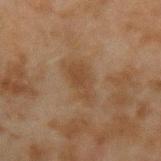The lesion was tiled from a total-body skin photograph and was not biopsied. A 15 mm close-up extracted from a 3D total-body photography capture. From the arm. The subject is a male in their mid-40s. Approximately 5 mm at its widest.